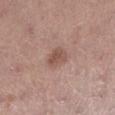Imaged during a routine full-body skin examination; the lesion was not biopsied and no histopathology is available. This is a white-light tile. The lesion's longest dimension is about 2.5 mm. Automated image analysis of the tile measured internal color variation of about 2.5 on a 0–10 scale and radial color variation of about 1. The software also gave a classifier nevus-likeness of about 40/100 and a detector confidence of about 100 out of 100 that the crop contains a lesion. A female subject, in their 60s. A region of skin cropped from a whole-body photographic capture, roughly 15 mm wide. The lesion is located on the right lower leg.